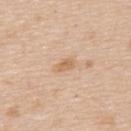{"biopsy_status": "not biopsied; imaged during a skin examination", "patient": {"sex": "female", "age_approx": 45}, "lighting": "white-light", "image": {"source": "total-body photography crop", "field_of_view_mm": 15}, "lesion_size": {"long_diameter_mm_approx": 3.0}, "site": "upper back"}